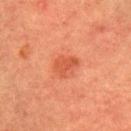Captured under cross-polarized illumination. Cropped from a whole-body photographic skin survey; the tile spans about 15 mm. About 2.5 mm across. The subject is a female in their mid- to late 50s. From the right upper arm.